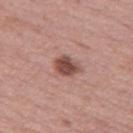| feature | finding |
|---|---|
| subject | female, approximately 55 years of age |
| acquisition | total-body-photography crop, ~15 mm field of view |
| body site | the left thigh |
| lighting | white-light illumination |